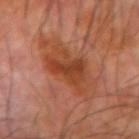The tile uses cross-polarized illumination. The lesion is located on the left forearm. Measured at roughly 7.5 mm in maximum diameter. Automated tile analysis of the lesion measured a shape eccentricity near 0.8 and two-axis asymmetry of about 0.3. It also reported a mean CIELAB color near L≈44 a*≈27 b*≈34. The analysis additionally found peripheral color asymmetry of about 1.5. And it measured a lesion-detection confidence of about 100/100. A male subject, aged around 70. A 15 mm close-up tile from a total-body photography series done for melanoma screening.